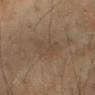The lesion is located on the right forearm. A 15 mm close-up tile from a total-body photography series done for melanoma screening. A female subject, aged 68 to 72. The total-body-photography lesion software estimated a shape-asymmetry score of about 0.25 (0 = symmetric). The software also gave an average lesion color of about L≈38 a*≈11 b*≈23 (CIELAB) and about 5 CIELAB-L* units darker than the surrounding skin. It also reported an automated nevus-likeness rating near 0 out of 100 and a detector confidence of about 70 out of 100 that the crop contains a lesion. Longest diameter approximately 5.5 mm.